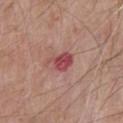notes: imaged on a skin check; not biopsied | body site: the chest | diameter: ~3.5 mm (longest diameter) | tile lighting: white-light illumination | image-analysis metrics: an area of roughly 6 mm² | imaging modality: total-body-photography crop, ~15 mm field of view | subject: male, aged 63–67.